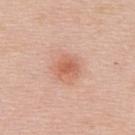| key | value |
|---|---|
| notes | catalogued during a skin exam; not biopsied |
| lighting | white-light |
| patient | female, aged 43–47 |
| location | the back |
| size | ≈3 mm |
| image source | ~15 mm crop, total-body skin-cancer survey |